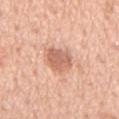notes — no biopsy performed (imaged during a skin exam) | imaging modality — ~15 mm crop, total-body skin-cancer survey | subject — male, aged 63–67 | site — the chest | lighting — white-light | size — ~4 mm (longest diameter).